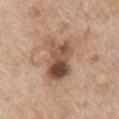follow-up — total-body-photography surveillance lesion; no biopsy
location — the right upper arm
tile lighting — white-light
image source — 15 mm crop, total-body photography
patient — female, roughly 75 years of age
lesion diameter — ≈5 mm